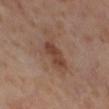<case>
  <biopsy_status>not biopsied; imaged during a skin examination</biopsy_status>
</case>